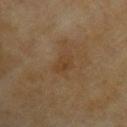Measured at roughly 3 mm in maximum diameter.
On the upper back.
This is a cross-polarized tile.
Cropped from a total-body skin-imaging series; the visible field is about 15 mm.
Automated image analysis of the tile measured a lesion area of about 4.5 mm². The software also gave a border-irregularity index near 2.5/10. And it measured an automated nevus-likeness rating near 0 out of 100.
A female subject roughly 60 years of age.